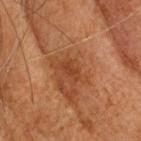No biopsy was performed on this lesion — it was imaged during a full skin examination and was not determined to be concerning. A 15 mm close-up extracted from a 3D total-body photography capture. A male patient about 65 years old. From the head or neck. The tile uses cross-polarized illumination. The recorded lesion diameter is about 2.5 mm.